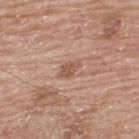Measured at roughly 2.5 mm in maximum diameter. A 15 mm close-up tile from a total-body photography series done for melanoma screening. A male patient, about 65 years old. Imaged with white-light lighting. Automated image analysis of the tile measured a lesion color around L≈54 a*≈21 b*≈28 in CIELAB, a lesion–skin lightness drop of about 9, and a normalized lesion–skin contrast near 6.5. The software also gave border irregularity of about 2.5 on a 0–10 scale, internal color variation of about 1.5 on a 0–10 scale, and peripheral color asymmetry of about 0.5. It also reported an automated nevus-likeness rating near 0 out of 100 and a detector confidence of about 100 out of 100 that the crop contains a lesion. Located on the upper back.